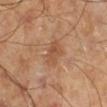Part of a total-body skin-imaging series; this lesion was reviewed on a skin check and was not flagged for biopsy.
On the right lower leg.
A lesion tile, about 15 mm wide, cut from a 3D total-body photograph.
The subject is a male aged around 45.